This is a white-light tile. A roughly 15 mm field-of-view crop from a total-body skin photograph. An algorithmic analysis of the crop reported a shape-asymmetry score of about 0.2 (0 = symmetric). The analysis additionally found a mean CIELAB color near L≈46 a*≈18 b*≈19, about 14 CIELAB-L* units darker than the surrounding skin, and a normalized border contrast of about 10. And it measured a border-irregularity rating of about 2.5/10, a within-lesion color-variation index near 6/10, and radial color variation of about 2. It also reported a classifier nevus-likeness of about 65/100 and a detector confidence of about 100 out of 100 that the crop contains a lesion. The lesion is on the right lower leg. Measured at roughly 4 mm in maximum diameter. A female subject roughly 40 years of age: 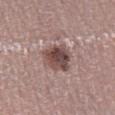On biopsy, histopathology showed a dysplastic (Clark) nevus — a benign lesion.